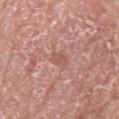workup — no biopsy performed (imaged during a skin exam) | tile lighting — white-light illumination | TBP lesion metrics — a lesion area of about 3 mm², a shape eccentricity near 0.75, and two-axis asymmetry of about 0.2; a detector confidence of about 100 out of 100 that the crop contains a lesion | subject — male, about 80 years old | body site — the right lower leg | imaging modality — ~15 mm tile from a whole-body skin photo.